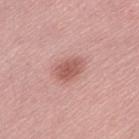Imaged during a routine full-body skin examination; the lesion was not biopsied and no histopathology is available.